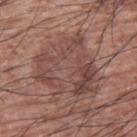{
  "biopsy_status": "not biopsied; imaged during a skin examination",
  "patient": {
    "sex": "male",
    "age_approx": 55
  },
  "lighting": "white-light",
  "image": {
    "source": "total-body photography crop",
    "field_of_view_mm": 15
  },
  "site": "left upper arm"
}